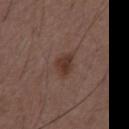Impression: Part of a total-body skin-imaging series; this lesion was reviewed on a skin check and was not flagged for biopsy. Image and clinical context: Automated tile analysis of the lesion measured an area of roughly 4.5 mm², a shape eccentricity near 0.6, and two-axis asymmetry of about 0.25. The software also gave a mean CIELAB color near L≈34 a*≈18 b*≈23 and roughly 9 lightness units darker than nearby skin. The recorded lesion diameter is about 2.5 mm. On the chest. A close-up tile cropped from a whole-body skin photograph, about 15 mm across. A male subject aged around 50. Imaged with white-light lighting.